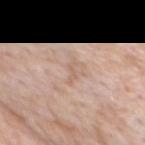Recorded during total-body skin imaging; not selected for excision or biopsy.
A male subject, roughly 80 years of age.
This is a white-light tile.
Longest diameter approximately 2.5 mm.
A 15 mm close-up tile from a total-body photography series done for melanoma screening.
The lesion is on the chest.
Automated image analysis of the tile measured internal color variation of about 0 on a 0–10 scale and radial color variation of about 0. It also reported a classifier nevus-likeness of about 0/100 and a detector confidence of about 100 out of 100 that the crop contains a lesion.